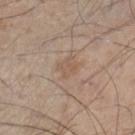<tbp_lesion>
  <biopsy_status>not biopsied; imaged during a skin examination</biopsy_status>
  <patient>
    <sex>male</sex>
    <age_approx>45</age_approx>
  </patient>
  <lesion_size>
    <long_diameter_mm_approx>3.0</long_diameter_mm_approx>
  </lesion_size>
  <automated_metrics>
    <area_mm2_approx>5.0</area_mm2_approx>
    <eccentricity>0.65</eccentricity>
    <cielab_L>56</cielab_L>
    <cielab_a>16</cielab_a>
    <cielab_b>28</cielab_b>
    <vs_skin_darker_L>6.0</vs_skin_darker_L>
    <border_irregularity_0_10>2.5</border_irregularity_0_10>
    <color_variation_0_10>2.5</color_variation_0_10>
    <peripheral_color_asymmetry>1.0</peripheral_color_asymmetry>
    <nevus_likeness_0_100>0</nevus_likeness_0_100>
    <lesion_detection_confidence_0_100>100</lesion_detection_confidence_0_100>
  </automated_metrics>
  <image>
    <source>total-body photography crop</source>
    <field_of_view_mm>15</field_of_view_mm>
  </image>
  <site>leg</site>
  <lighting>white-light</lighting>
</tbp_lesion>